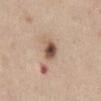Impression: The lesion was photographed on a routine skin check and not biopsied; there is no pathology result. Acquisition and patient details: The tile uses white-light illumination. Longest diameter approximately 3.5 mm. A region of skin cropped from a whole-body photographic capture, roughly 15 mm wide. A female subject, about 40 years old. Located on the abdomen. The lesion-visualizer software estimated a lesion color around L≈53 a*≈17 b*≈28 in CIELAB, a lesion–skin lightness drop of about 15, and a lesion-to-skin contrast of about 10 (normalized; higher = more distinct). The analysis additionally found a classifier nevus-likeness of about 80/100 and lesion-presence confidence of about 100/100.